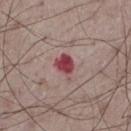The lesion was photographed on a routine skin check and not biopsied; there is no pathology result.
A male patient, roughly 75 years of age.
Automated tile analysis of the lesion measured an outline eccentricity of about 0.65 (0 = round, 1 = elongated) and two-axis asymmetry of about 0.25. It also reported an average lesion color of about L≈44 a*≈31 b*≈18 (CIELAB), a lesion–skin lightness drop of about 15, and a normalized lesion–skin contrast near 11. It also reported a border-irregularity rating of about 2.5/10, a color-variation rating of about 3/10, and radial color variation of about 1. The software also gave an automated nevus-likeness rating near 0 out of 100 and a lesion-detection confidence of about 100/100.
On the left thigh.
A 15 mm crop from a total-body photograph taken for skin-cancer surveillance.
This is a white-light tile.
About 3 mm across.